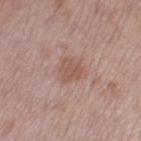  patient:
    sex: female
    age_approx: 50
  lighting: white-light
  site: left thigh
  image:
    source: total-body photography crop
    field_of_view_mm: 15
  automated_metrics:
    cielab_L: 54
    cielab_a: 20
    cielab_b: 25
    vs_skin_contrast_norm: 6.0
  lesion_size:
    long_diameter_mm_approx: 2.5